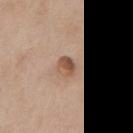Impression: Imaged during a routine full-body skin examination; the lesion was not biopsied and no histopathology is available. Context: The recorded lesion diameter is about 2.5 mm. Cropped from a whole-body photographic skin survey; the tile spans about 15 mm. A male subject, aged 58 to 62. On the chest.